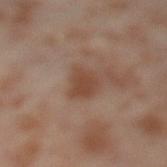workup: catalogued during a skin exam; not biopsied | body site: the left thigh | TBP lesion metrics: an eccentricity of roughly 0.7 and two-axis asymmetry of about 0.3; a within-lesion color-variation index near 1.5/10 and peripheral color asymmetry of about 0.5; a classifier nevus-likeness of about 0/100 and lesion-presence confidence of about 100/100 | patient: female, aged 53–57 | imaging modality: 15 mm crop, total-body photography.